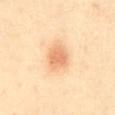{
  "biopsy_status": "not biopsied; imaged during a skin examination",
  "lighting": "cross-polarized",
  "lesion_size": {
    "long_diameter_mm_approx": 3.5
  },
  "patient": {
    "sex": "female",
    "age_approx": 40
  },
  "site": "mid back",
  "image": {
    "source": "total-body photography crop",
    "field_of_view_mm": 15
  }
}